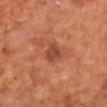Clinical impression:
Part of a total-body skin-imaging series; this lesion was reviewed on a skin check and was not flagged for biopsy.
Image and clinical context:
This is a cross-polarized tile. On the upper back. Automated image analysis of the tile measured a shape eccentricity near 0.75 and a shape-asymmetry score of about 0.3 (0 = symmetric). The analysis additionally found a lesion color around L≈43 a*≈28 b*≈32 in CIELAB, a lesion–skin lightness drop of about 9, and a lesion-to-skin contrast of about 7.5 (normalized; higher = more distinct). A male patient, in their 60s. A roughly 15 mm field-of-view crop from a total-body skin photograph.